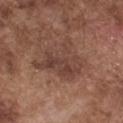Clinical impression:
Captured during whole-body skin photography for melanoma surveillance; the lesion was not biopsied.
Image and clinical context:
Longest diameter approximately 5.5 mm. Located on the chest. This image is a 15 mm lesion crop taken from a total-body photograph. The patient is a male aged approximately 75. The total-body-photography lesion software estimated a border-irregularity rating of about 6/10 and peripheral color asymmetry of about 1.5. The analysis additionally found a detector confidence of about 100 out of 100 that the crop contains a lesion. The tile uses white-light illumination.